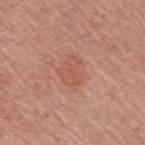Impression:
This lesion was catalogued during total-body skin photography and was not selected for biopsy.
Image and clinical context:
A lesion tile, about 15 mm wide, cut from a 3D total-body photograph. The subject is a female aged 63 to 67. The lesion is located on the right upper arm.